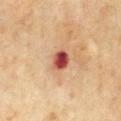The lesion was photographed on a routine skin check and not biopsied; there is no pathology result. A male patient, aged around 70. Automated image analysis of the tile measured an eccentricity of roughly 0.6. The software also gave border irregularity of about 2 on a 0–10 scale, a within-lesion color-variation index near 5.5/10, and peripheral color asymmetry of about 1.5. A 15 mm close-up tile from a total-body photography series done for melanoma screening. The tile uses cross-polarized illumination. Located on the chest.